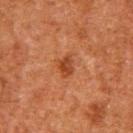Q: Is there a histopathology result?
A: catalogued during a skin exam; not biopsied
Q: How large is the lesion?
A: ≈2.5 mm
Q: What are the patient's age and sex?
A: male, roughly 80 years of age
Q: What kind of image is this?
A: ~15 mm crop, total-body skin-cancer survey
Q: Where on the body is the lesion?
A: the mid back
Q: Automated lesion metrics?
A: an area of roughly 4.5 mm² and a symmetry-axis asymmetry near 0.35; a mean CIELAB color near L≈35 a*≈24 b*≈32, a lesion–skin lightness drop of about 8, and a normalized lesion–skin contrast near 7.5; a classifier nevus-likeness of about 80/100 and a lesion-detection confidence of about 100/100